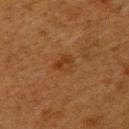notes = no biopsy performed (imaged during a skin exam) | image source = 15 mm crop, total-body photography | subject = female, aged approximately 40 | diameter = ≈2.5 mm | site = the upper back.